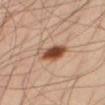Part of a total-body skin-imaging series; this lesion was reviewed on a skin check and was not flagged for biopsy.
Captured under cross-polarized illumination.
Cropped from a total-body skin-imaging series; the visible field is about 15 mm.
The subject is a male aged approximately 35.
Approximately 4 mm at its widest.
From the right thigh.